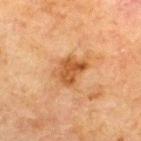workup: no biopsy performed (imaged during a skin exam) | TBP lesion metrics: an average lesion color of about L≈45 a*≈22 b*≈36 (CIELAB), about 10 CIELAB-L* units darker than the surrounding skin, and a normalized border contrast of about 8.5; border irregularity of about 3 on a 0–10 scale and a peripheral color-asymmetry measure near 1 | illumination: cross-polarized | lesion diameter: ~3.5 mm (longest diameter) | anatomic site: the upper back | imaging modality: 15 mm crop, total-body photography | subject: male, aged approximately 70.